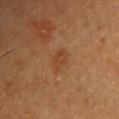<case>
<biopsy_status>not biopsied; imaged during a skin examination</biopsy_status>
<lesion_size>
  <long_diameter_mm_approx>3.0</long_diameter_mm_approx>
</lesion_size>
<lighting>cross-polarized</lighting>
<automated_metrics>
  <cielab_L>34</cielab_L>
  <cielab_a>17</cielab_a>
  <cielab_b>29</cielab_b>
  <vs_skin_darker_L>5.0</vs_skin_darker_L>
  <border_irregularity_0_10>2.5</border_irregularity_0_10>
  <color_variation_0_10>2.0</color_variation_0_10>
  <peripheral_color_asymmetry>0.5</peripheral_color_asymmetry>
</automated_metrics>
<patient>
  <sex>female</sex>
  <age_approx>55</age_approx>
</patient>
<site>upper back</site>
<image>
  <source>total-body photography crop</source>
  <field_of_view_mm>15</field_of_view_mm>
</image>
</case>